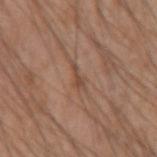Clinical summary: The lesion is located on the left upper arm. A male subject, approximately 50 years of age. About 3 mm across. A close-up tile cropped from a whole-body skin photograph, about 15 mm across.